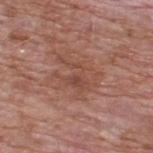Q: Is there a histopathology result?
A: imaged on a skin check; not biopsied
Q: What lighting was used for the tile?
A: white-light
Q: Where on the body is the lesion?
A: the upper back
Q: Automated lesion metrics?
A: a footprint of about 12 mm², an outline eccentricity of about 0.7 (0 = round, 1 = elongated), and a shape-asymmetry score of about 0.45 (0 = symmetric); a classifier nevus-likeness of about 0/100 and a lesion-detection confidence of about 100/100
Q: What are the patient's age and sex?
A: male, approximately 60 years of age
Q: What is the imaging modality?
A: total-body-photography crop, ~15 mm field of view
Q: Lesion size?
A: ≈5 mm